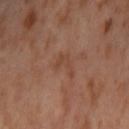Imaged during a routine full-body skin examination; the lesion was not biopsied and no histopathology is available.
The total-body-photography lesion software estimated an average lesion color of about L≈44 a*≈21 b*≈30 (CIELAB). The analysis additionally found border irregularity of about 8 on a 0–10 scale and a peripheral color-asymmetry measure near 0. It also reported an automated nevus-likeness rating near 0 out of 100 and lesion-presence confidence of about 100/100.
A female subject, about 55 years old.
The lesion is on the left thigh.
Cropped from a total-body skin-imaging series; the visible field is about 15 mm.
Approximately 3.5 mm at its widest.